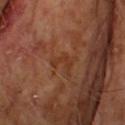acquisition = 15 mm crop, total-body photography; tile lighting = cross-polarized illumination; body site = the head or neck; patient = male, aged around 65; lesion diameter = about 2.5 mm; TBP lesion metrics = an automated nevus-likeness rating near 0 out of 100 and lesion-presence confidence of about 100/100.